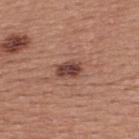Clinical impression:
Recorded during total-body skin imaging; not selected for excision or biopsy.
Image and clinical context:
From the upper back. The recorded lesion diameter is about 3 mm. The tile uses white-light illumination. A region of skin cropped from a whole-body photographic capture, roughly 15 mm wide. The subject is a male aged 38–42.